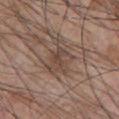  biopsy_status: not biopsied; imaged during a skin examination
  image:
    source: total-body photography crop
    field_of_view_mm: 15
  lighting: white-light
  patient:
    sex: male
    age_approx: 70
  site: front of the torso